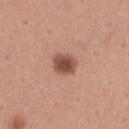This lesion was catalogued during total-body skin photography and was not selected for biopsy. A female patient, aged 28–32. Automated image analysis of the tile measured an average lesion color of about L≈50 a*≈23 b*≈28 (CIELAB), roughly 14 lightness units darker than nearby skin, and a normalized lesion–skin contrast near 9.5. The analysis additionally found a classifier nevus-likeness of about 100/100 and a lesion-detection confidence of about 100/100. Cropped from a total-body skin-imaging series; the visible field is about 15 mm. About 3 mm across. Imaged with white-light lighting. From the right thigh.